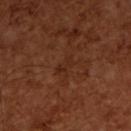Clinical impression: The lesion was photographed on a routine skin check and not biopsied; there is no pathology result. Acquisition and patient details: The tile uses cross-polarized illumination. A male subject aged 63 to 67. Cropped from a total-body skin-imaging series; the visible field is about 15 mm. Automated tile analysis of the lesion measured a lesion area of about 2.5 mm², a shape eccentricity near 0.85, and a symmetry-axis asymmetry near 0.45. The software also gave about 4 CIELAB-L* units darker than the surrounding skin and a normalized border contrast of about 4.5. It also reported a border-irregularity rating of about 5.5/10, internal color variation of about 0 on a 0–10 scale, and a peripheral color-asymmetry measure near 0. The lesion's longest dimension is about 2.5 mm.